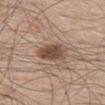Q: Was this lesion biopsied?
A: total-body-photography surveillance lesion; no biopsy
Q: Where on the body is the lesion?
A: the leg
Q: Who is the patient?
A: male, approximately 60 years of age
Q: What is the imaging modality?
A: ~15 mm tile from a whole-body skin photo